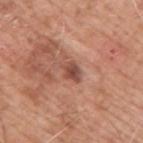lighting: white-light illumination | acquisition: ~15 mm crop, total-body skin-cancer survey | subject: male, approximately 60 years of age | lesion size: ≈2.5 mm | site: the arm | TBP lesion metrics: a mean CIELAB color near L≈48 a*≈24 b*≈28, roughly 12 lightness units darker than nearby skin, and a normalized lesion–skin contrast near 8.5; border irregularity of about 2.5 on a 0–10 scale and a within-lesion color-variation index near 4.5/10.